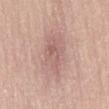Part of a total-body skin-imaging series; this lesion was reviewed on a skin check and was not flagged for biopsy.
A roughly 15 mm field-of-view crop from a total-body skin photograph.
The patient is a male aged 53 to 57.